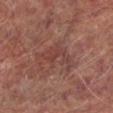Case summary:
* biopsy status: catalogued during a skin exam; not biopsied
* size: ≈5.5 mm
* lighting: cross-polarized
* location: the leg
* patient: male, aged around 65
* imaging modality: 15 mm crop, total-body photography